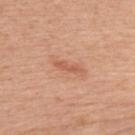• notes: catalogued during a skin exam; not biopsied
• lighting: white-light
• site: the upper back
• size: ~3 mm (longest diameter)
• imaging modality: total-body-photography crop, ~15 mm field of view
• patient: male, aged 48 to 52